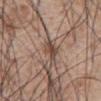Captured during whole-body skin photography for melanoma surveillance; the lesion was not biopsied.
A male subject approximately 60 years of age.
Automated image analysis of the tile measured border irregularity of about 3 on a 0–10 scale, a within-lesion color-variation index near 4.5/10, and a peripheral color-asymmetry measure near 1. It also reported an automated nevus-likeness rating near 40 out of 100 and lesion-presence confidence of about 100/100.
A roughly 15 mm field-of-view crop from a total-body skin photograph.
The lesion's longest dimension is about 3 mm.
The lesion is located on the front of the torso.